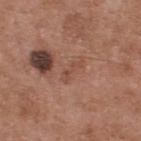Case summary:
- biopsy status — total-body-photography surveillance lesion; no biopsy
- diameter — ~3 mm (longest diameter)
- anatomic site — the back
- image — total-body-photography crop, ~15 mm field of view
- lighting — white-light
- subject — male, in their mid- to late 50s